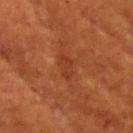Impression: Recorded during total-body skin imaging; not selected for excision or biopsy.